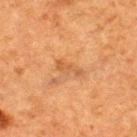| field | value |
|---|---|
| follow-up | total-body-photography surveillance lesion; no biopsy |
| patient | male, aged around 50 |
| lesion size | about 3 mm |
| anatomic site | the upper back |
| acquisition | total-body-photography crop, ~15 mm field of view |
| illumination | cross-polarized illumination |
| automated metrics | a lesion area of about 3 mm², an eccentricity of roughly 0.95, and two-axis asymmetry of about 0.45; a mean CIELAB color near L≈46 a*≈21 b*≈35 and a normalized border contrast of about 5.5 |